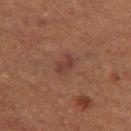This lesion was catalogued during total-body skin photography and was not selected for biopsy. The patient is a male aged around 75. A 15 mm crop from a total-body photograph taken for skin-cancer surveillance. An algorithmic analysis of the crop reported a lesion color around L≈30 a*≈18 b*≈20 in CIELAB, roughly 6 lightness units darker than nearby skin, and a normalized lesion–skin contrast near 6.5. On the left thigh.